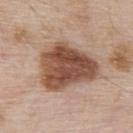Part of a total-body skin-imaging series; this lesion was reviewed on a skin check and was not flagged for biopsy. The recorded lesion diameter is about 7 mm. A 15 mm close-up tile from a total-body photography series done for melanoma screening. The subject is a male aged 53 to 57. The tile uses white-light illumination. From the upper back. An algorithmic analysis of the crop reported an area of roughly 29 mm², an outline eccentricity of about 0.65 (0 = round, 1 = elongated), and a symmetry-axis asymmetry near 0.35. The software also gave a mean CIELAB color near L≈50 a*≈20 b*≈28, a lesion–skin lightness drop of about 16, and a normalized border contrast of about 11. It also reported a classifier nevus-likeness of about 95/100 and a detector confidence of about 100 out of 100 that the crop contains a lesion.